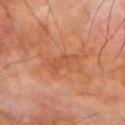Clinical impression:
This lesion was catalogued during total-body skin photography and was not selected for biopsy.
Clinical summary:
On the left forearm. The subject is a male roughly 70 years of age. A 15 mm close-up extracted from a 3D total-body photography capture. This is a cross-polarized tile. The recorded lesion diameter is about 4 mm.